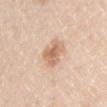The lesion was photographed on a routine skin check and not biopsied; there is no pathology result. A 15 mm crop from a total-body photograph taken for skin-cancer surveillance. The lesion is on the right upper arm. Imaged with white-light lighting. A male subject, approximately 45 years of age. The recorded lesion diameter is about 3.5 mm.